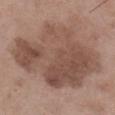This lesion was catalogued during total-body skin photography and was not selected for biopsy.
The subject is a male roughly 50 years of age.
This image is a 15 mm lesion crop taken from a total-body photograph.
Located on the right lower leg.